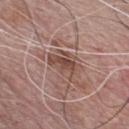Part of a total-body skin-imaging series; this lesion was reviewed on a skin check and was not flagged for biopsy.
On the chest.
Cropped from a whole-body photographic skin survey; the tile spans about 15 mm.
A male subject, in their mid- to late 70s.
About 4 mm across.
This is a white-light tile.
Automated tile analysis of the lesion measured a footprint of about 8 mm². The analysis additionally found a lesion color around L≈48 a*≈19 b*≈24 in CIELAB, roughly 10 lightness units darker than nearby skin, and a normalized border contrast of about 7.5.